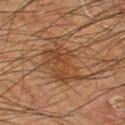follow-up — no biopsy performed (imaged during a skin exam)
subject — male, about 65 years old
image — total-body-photography crop, ~15 mm field of view
location — the left thigh